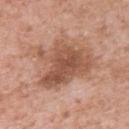The lesion was photographed on a routine skin check and not biopsied; there is no pathology result. The subject is a female in their 50s. This image is a 15 mm lesion crop taken from a total-body photograph. This is a white-light tile. About 7 mm across. The lesion-visualizer software estimated a lesion area of about 24 mm² and two-axis asymmetry of about 0.4. The analysis additionally found lesion-presence confidence of about 100/100. On the upper back.